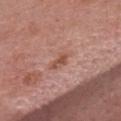notes = total-body-photography surveillance lesion; no biopsy | illumination = white-light illumination | location = the head or neck | patient = male, aged approximately 55 | image source = total-body-photography crop, ~15 mm field of view.